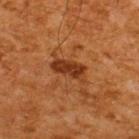Notes:
• biopsy status — no biopsy performed (imaged during a skin exam)
• anatomic site — the back
• image-analysis metrics — a shape-asymmetry score of about 0.25 (0 = symmetric); roughly 10 lightness units darker than nearby skin; border irregularity of about 3.5 on a 0–10 scale and internal color variation of about 2 on a 0–10 scale; a nevus-likeness score of about 5/100 and a lesion-detection confidence of about 100/100
• size — ≈4.5 mm
• image source — ~15 mm crop, total-body skin-cancer survey
• lighting — cross-polarized illumination
• subject — male, approximately 65 years of age